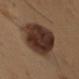Impression:
No biopsy was performed on this lesion — it was imaged during a full skin examination and was not determined to be concerning.
Background:
This image is a 15 mm lesion crop taken from a total-body photograph. The patient is a male approximately 50 years of age. From the left upper arm.